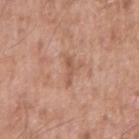Clinical impression: Imaged during a routine full-body skin examination; the lesion was not biopsied and no histopathology is available. Acquisition and patient details: This image is a 15 mm lesion crop taken from a total-body photograph. The lesion is located on the right upper arm. A male subject, aged approximately 55.